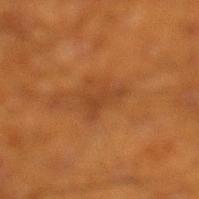Recorded during total-body skin imaging; not selected for excision or biopsy. The lesion is located on the left lower leg. Imaged with cross-polarized lighting. A 15 mm close-up extracted from a 3D total-body photography capture. A male subject in their 60s.